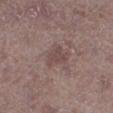A male subject in their 70s. The recorded lesion diameter is about 3 mm. Imaged with white-light lighting. A lesion tile, about 15 mm wide, cut from a 3D total-body photograph. The lesion is located on the left lower leg.